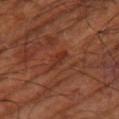Findings:
* workup: no biopsy performed (imaged during a skin exam)
* image: total-body-photography crop, ~15 mm field of view
* image-analysis metrics: a footprint of about 2.5 mm²
* illumination: cross-polarized illumination
* body site: the leg
* patient: aged 63 to 67